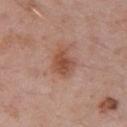<record>
  <biopsy_status>not biopsied; imaged during a skin examination</biopsy_status>
  <lighting>white-light</lighting>
  <image>
    <source>total-body photography crop</source>
    <field_of_view_mm>15</field_of_view_mm>
  </image>
  <lesion_size>
    <long_diameter_mm_approx>3.5</long_diameter_mm_approx>
  </lesion_size>
  <site>right upper arm</site>
  <automated_metrics>
    <border_irregularity_0_10>2.5</border_irregularity_0_10>
    <color_variation_0_10>3.5</color_variation_0_10>
    <peripheral_color_asymmetry>1.0</peripheral_color_asymmetry>
    <lesion_detection_confidence_0_100>100</lesion_detection_confidence_0_100>
  </automated_metrics>
  <patient>
    <sex>male</sex>
    <age_approx>55</age_approx>
  </patient>
</record>